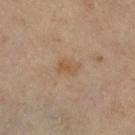Assessment:
No biopsy was performed on this lesion — it was imaged during a full skin examination and was not determined to be concerning.
Context:
This image is a 15 mm lesion crop taken from a total-body photograph. On the left leg. A female patient, roughly 65 years of age.